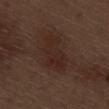– biopsy status — total-body-photography surveillance lesion; no biopsy
– imaging modality — ~15 mm tile from a whole-body skin photo
– image-analysis metrics — a lesion color around L≈25 a*≈17 b*≈21 in CIELAB, roughly 5 lightness units darker than nearby skin, and a lesion-to-skin contrast of about 6 (normalized; higher = more distinct); a border-irregularity index near 3.5/10; a classifier nevus-likeness of about 30/100
– tile lighting — white-light
– patient — male, aged around 70
– body site — the right thigh
– size — ~5.5 mm (longest diameter)